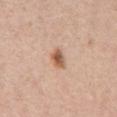Q: Where on the body is the lesion?
A: the abdomen
Q: What is the imaging modality?
A: ~15 mm crop, total-body skin-cancer survey
Q: Lesion size?
A: ≈2.5 mm
Q: Illumination type?
A: white-light
Q: What are the patient's age and sex?
A: female, in their 60s